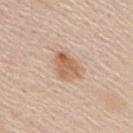The lesion was tiled from a total-body skin photograph and was not biopsied. This is a white-light tile. The patient is a female aged approximately 60. Approximately 3.5 mm at its widest. Cropped from a total-body skin-imaging series; the visible field is about 15 mm. From the upper back.